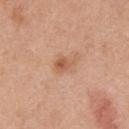Assessment:
No biopsy was performed on this lesion — it was imaged during a full skin examination and was not determined to be concerning.
Clinical summary:
The lesion is located on the chest. This image is a 15 mm lesion crop taken from a total-body photograph. A male patient, aged around 70.